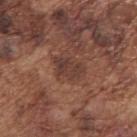Acquisition and patient details: The lesion's longest dimension is about 4 mm. A 15 mm close-up extracted from a 3D total-body photography capture. Located on the right upper arm. A male subject in their mid-70s.